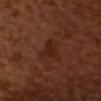notes — imaged on a skin check; not biopsied
subject — male, aged 53–57
lesion size — ~3.5 mm (longest diameter)
body site — the head or neck
lighting — cross-polarized
image source — total-body-photography crop, ~15 mm field of view